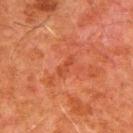Q: Was a biopsy performed?
A: total-body-photography surveillance lesion; no biopsy
Q: Patient demographics?
A: male, aged approximately 80
Q: What did automated image analysis measure?
A: an area of roughly 2 mm², an outline eccentricity of about 0.95 (0 = round, 1 = elongated), and a shape-asymmetry score of about 0.5 (0 = symmetric); a border-irregularity rating of about 7/10, internal color variation of about 0 on a 0–10 scale, and radial color variation of about 0
Q: What is the anatomic site?
A: the back
Q: Illumination type?
A: cross-polarized
Q: What is the lesion's diameter?
A: ~2.5 mm (longest diameter)
Q: How was this image acquired?
A: total-body-photography crop, ~15 mm field of view